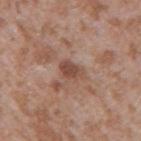Assessment: The lesion was tiled from a total-body skin photograph and was not biopsied. Acquisition and patient details: From the right upper arm. A 15 mm close-up extracted from a 3D total-body photography capture. The patient is a male in their mid-40s. Captured under white-light illumination.